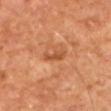Impression: This lesion was catalogued during total-body skin photography and was not selected for biopsy. Image and clinical context: The total-body-photography lesion software estimated an area of roughly 3 mm², an outline eccentricity of about 0.9 (0 = round, 1 = elongated), and a shape-asymmetry score of about 0.35 (0 = symmetric). The software also gave a border-irregularity rating of about 4.5/10, a color-variation rating of about 0/10, and a peripheral color-asymmetry measure near 0. And it measured a lesion-detection confidence of about 100/100. A male subject, aged around 65. This image is a 15 mm lesion crop taken from a total-body photograph. The lesion is located on the left upper arm.